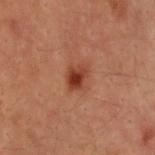Case summary:
• notes — no biopsy performed (imaged during a skin exam)
• site — the back
• lesion size — ≈3 mm
• subject — male, aged approximately 30
• automated lesion analysis — an outline eccentricity of about 0.7 (0 = round, 1 = elongated)
• image — ~15 mm tile from a whole-body skin photo